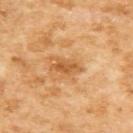notes: total-body-photography surveillance lesion; no biopsy | image source: total-body-photography crop, ~15 mm field of view | lesion size: ~4 mm (longest diameter) | body site: the upper back | subject: female, aged around 60 | automated metrics: an area of roughly 5 mm², a shape eccentricity near 0.9, and a shape-asymmetry score of about 0.25 (0 = symmetric); an average lesion color of about L≈57 a*≈24 b*≈44 (CIELAB), roughly 10 lightness units darker than nearby skin, and a lesion-to-skin contrast of about 7 (normalized; higher = more distinct); a nevus-likeness score of about 0/100 and lesion-presence confidence of about 100/100.